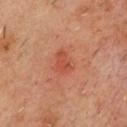Clinical impression:
The lesion was photographed on a routine skin check and not biopsied; there is no pathology result.
Image and clinical context:
A lesion tile, about 15 mm wide, cut from a 3D total-body photograph. The tile uses cross-polarized illumination. A male patient aged 58–62. The recorded lesion diameter is about 3 mm. From the front of the torso.